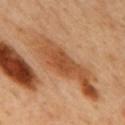<tbp_lesion>
  <biopsy_status>not biopsied; imaged during a skin examination</biopsy_status>
  <lesion_size>
    <long_diameter_mm_approx>3.0</long_diameter_mm_approx>
  </lesion_size>
  <automated_metrics>
    <area_mm2_approx>5.5</area_mm2_approx>
    <eccentricity>0.8</eccentricity>
    <shape_asymmetry>0.15</shape_asymmetry>
    <cielab_L>48</cielab_L>
    <cielab_a>26</cielab_a>
    <cielab_b>38</cielab_b>
    <vs_skin_darker_L>9.0</vs_skin_darker_L>
    <border_irregularity_0_10>1.5</border_irregularity_0_10>
    <color_variation_0_10>2.0</color_variation_0_10>
    <nevus_likeness_0_100>0</nevus_likeness_0_100>
  </automated_metrics>
  <image>
    <source>total-body photography crop</source>
    <field_of_view_mm>15</field_of_view_mm>
  </image>
  <patient>
    <sex>male</sex>
    <age_approx>50</age_approx>
  </patient>
  <lighting>cross-polarized</lighting>
  <site>back</site>
</tbp_lesion>